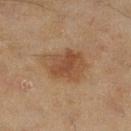Recorded during total-body skin imaging; not selected for excision or biopsy. A lesion tile, about 15 mm wide, cut from a 3D total-body photograph. The lesion is on the right lower leg. Measured at roughly 6 mm in maximum diameter. The patient is a female roughly 60 years of age. Captured under cross-polarized illumination.